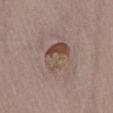biopsy status: imaged on a skin check; not biopsied
patient: male, aged around 75
diameter: ≈4.5 mm
automated lesion analysis: an area of roughly 9.5 mm², an eccentricity of roughly 0.85, and two-axis asymmetry of about 0.35; an automated nevus-likeness rating near 25 out of 100 and a lesion-detection confidence of about 95/100
anatomic site: the lower back
image source: total-body-photography crop, ~15 mm field of view